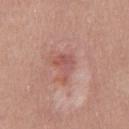| field | value |
|---|---|
| biopsy status | imaged on a skin check; not biopsied |